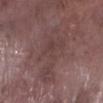Notes:
– biopsy status · catalogued during a skin exam; not biopsied
– tile lighting · white-light
– TBP lesion metrics · an outline eccentricity of about 0.9 (0 = round, 1 = elongated) and a shape-asymmetry score of about 0.45 (0 = symmetric); border irregularity of about 6 on a 0–10 scale, internal color variation of about 3 on a 0–10 scale, and peripheral color asymmetry of about 1
– location · the right lower leg
– imaging modality · ~15 mm crop, total-body skin-cancer survey
– size · about 8 mm
– patient · male, in their mid- to late 70s